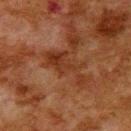Case summary:
• follow-up · total-body-photography surveillance lesion; no biopsy
• image source · total-body-photography crop, ~15 mm field of view
• diameter · ≈6 mm
• patient · male, aged around 80
• site · the upper back
• lighting · cross-polarized illumination